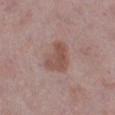biopsy status — catalogued during a skin exam; not biopsied
lesion diameter — ≈4 mm
location — the left lower leg
subject — female, aged 58–62
image source — 15 mm crop, total-body photography